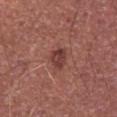* follow-up — imaged on a skin check; not biopsied
* image source — 15 mm crop, total-body photography
* site — the abdomen
* automated metrics — a footprint of about 4.5 mm²; a lesion color around L≈39 a*≈25 b*≈23 in CIELAB and a lesion–skin lightness drop of about 10; a color-variation rating of about 3/10 and a peripheral color-asymmetry measure near 1
* lesion size — ≈2.5 mm
* subject — male, aged approximately 65
* tile lighting — white-light illumination